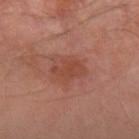Part of a total-body skin-imaging series; this lesion was reviewed on a skin check and was not flagged for biopsy. The tile uses cross-polarized illumination. The lesion is on the left forearm. Automated tile analysis of the lesion measured an outline eccentricity of about 0.85 (0 = round, 1 = elongated) and a symmetry-axis asymmetry near 0.3. And it measured an average lesion color of about L≈43 a*≈25 b*≈28 (CIELAB) and about 7 CIELAB-L* units darker than the surrounding skin. The software also gave a border-irregularity rating of about 3.5/10 and a within-lesion color-variation index near 1.5/10. The analysis additionally found an automated nevus-likeness rating near 5 out of 100 and lesion-presence confidence of about 100/100. A male patient, in their mid-60s. A 15 mm close-up extracted from a 3D total-body photography capture. Approximately 4 mm at its widest.